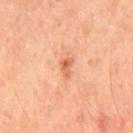Clinical impression: No biopsy was performed on this lesion — it was imaged during a full skin examination and was not determined to be concerning. Clinical summary: A close-up tile cropped from a whole-body skin photograph, about 15 mm across. The total-body-photography lesion software estimated an area of roughly 2.5 mm² and two-axis asymmetry of about 0.5. The software also gave a mean CIELAB color near L≈60 a*≈28 b*≈38 and a lesion–skin lightness drop of about 10. The analysis additionally found a border-irregularity index near 4.5/10, a within-lesion color-variation index near 2.5/10, and peripheral color asymmetry of about 1. Longest diameter approximately 2.5 mm. Imaged with cross-polarized lighting. The patient is a male approximately 45 years of age. The lesion is on the mid back.